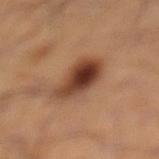{"biopsy_status": "not biopsied; imaged during a skin examination", "image": {"source": "total-body photography crop", "field_of_view_mm": 15}, "lesion_size": {"long_diameter_mm_approx": 4.5}, "patient": {"sex": "male", "age_approx": 50}, "site": "leg"}